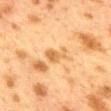Q: Was this lesion biopsied?
A: imaged on a skin check; not biopsied
Q: How large is the lesion?
A: ~3.5 mm (longest diameter)
Q: What is the imaging modality?
A: 15 mm crop, total-body photography
Q: Where on the body is the lesion?
A: the mid back
Q: What did automated image analysis measure?
A: a lesion area of about 5 mm², a shape eccentricity near 0.85, and a shape-asymmetry score of about 0.45 (0 = symmetric); a lesion color around L≈56 a*≈19 b*≈39 in CIELAB and a lesion-to-skin contrast of about 6.5 (normalized; higher = more distinct); a border-irregularity rating of about 5/10 and a within-lesion color-variation index near 2/10
Q: Patient demographics?
A: female, in their 40s
Q: How was the tile lit?
A: cross-polarized